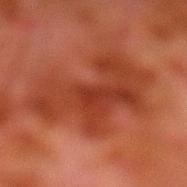image source=total-body-photography crop, ~15 mm field of view; anatomic site=the leg; patient=male, aged 78 to 82; illumination=cross-polarized illumination.